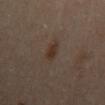Impression:
The lesion was tiled from a total-body skin photograph and was not biopsied.
Context:
Captured under cross-polarized illumination. Approximately 3 mm at its widest. A male subject, aged 63 to 67. Automated tile analysis of the lesion measured a lesion area of about 4 mm², an outline eccentricity of about 0.9 (0 = round, 1 = elongated), and a shape-asymmetry score of about 0.25 (0 = symmetric). The software also gave an average lesion color of about L≈32 a*≈14 b*≈23 (CIELAB) and a normalized border contrast of about 7.5. The software also gave a detector confidence of about 100 out of 100 that the crop contains a lesion. On the back. A lesion tile, about 15 mm wide, cut from a 3D total-body photograph.